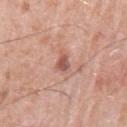  biopsy_status: not biopsied; imaged during a skin examination
  site: arm
  patient:
    sex: male
    age_approx: 80
  lesion_size:
    long_diameter_mm_approx: 2.5
  lighting: white-light
  image:
    source: total-body photography crop
    field_of_view_mm: 15